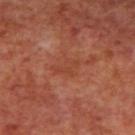workup: catalogued during a skin exam; not biopsied
site: the mid back
subject: male, aged approximately 70
tile lighting: cross-polarized illumination
acquisition: total-body-photography crop, ~15 mm field of view